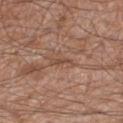- follow-up — imaged on a skin check; not biopsied
- size — ≈2.5 mm
- image source — ~15 mm tile from a whole-body skin photo
- location — the right thigh
- subject — male, about 55 years old
- tile lighting — white-light